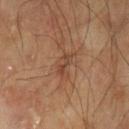This lesion was catalogued during total-body skin photography and was not selected for biopsy. The lesion is located on the left upper arm. Captured under cross-polarized illumination. A lesion tile, about 15 mm wide, cut from a 3D total-body photograph. The lesion-visualizer software estimated a lesion color around L≈36 a*≈18 b*≈26 in CIELAB and a lesion–skin lightness drop of about 7. It also reported a border-irregularity index near 5/10, internal color variation of about 0 on a 0–10 scale, and a peripheral color-asymmetry measure near 0. A male patient aged 73 to 77. The recorded lesion diameter is about 2.5 mm.